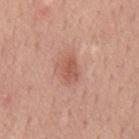Recorded during total-body skin imaging; not selected for excision or biopsy. The patient is a male roughly 50 years of age. A 15 mm close-up extracted from a 3D total-body photography capture. The lesion is on the mid back. Approximately 3 mm at its widest. This is a white-light tile.